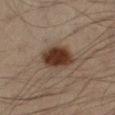Captured during whole-body skin photography for melanoma surveillance; the lesion was not biopsied. An algorithmic analysis of the crop reported an eccentricity of roughly 0.7 and two-axis asymmetry of about 0.2. The software also gave a border-irregularity index near 1.5/10, a within-lesion color-variation index near 4/10, and a peripheral color-asymmetry measure near 1. It also reported lesion-presence confidence of about 100/100. A male subject, in their mid- to late 30s. Located on the left lower leg. The tile uses cross-polarized illumination. A region of skin cropped from a whole-body photographic capture, roughly 15 mm wide.